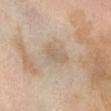Impression: This lesion was catalogued during total-body skin photography and was not selected for biopsy. Acquisition and patient details: Longest diameter approximately 3 mm. Imaged with cross-polarized lighting. A male patient, in their mid-50s. From the right forearm. A region of skin cropped from a whole-body photographic capture, roughly 15 mm wide.